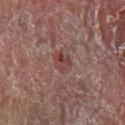The lesion was photographed on a routine skin check and not biopsied; there is no pathology result. A roughly 15 mm field-of-view crop from a total-body skin photograph. Longest diameter approximately 3 mm. Captured under cross-polarized illumination. A male subject, in their mid- to late 50s. From the left lower leg. The lesion-visualizer software estimated a lesion area of about 5 mm² and a symmetry-axis asymmetry near 0.2. The analysis additionally found a lesion color around L≈36 a*≈20 b*≈19 in CIELAB and roughly 7 lightness units darker than nearby skin. The software also gave a color-variation rating of about 5.5/10 and radial color variation of about 2. And it measured a nevus-likeness score of about 0/100.